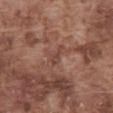biopsy_status: not biopsied; imaged during a skin examination
automated_metrics:
  area_mm2_approx: 3.0
  eccentricity: 0.9
  shape_asymmetry: 0.5
  border_irregularity_0_10: 6.0
  color_variation_0_10: 1.0
  peripheral_color_asymmetry: 0.5
  nevus_likeness_0_100: 0
  lesion_detection_confidence_0_100: 75
patient:
  sex: male
  age_approx: 75
image:
  source: total-body photography crop
  field_of_view_mm: 15
site: abdomen
lesion_size:
  long_diameter_mm_approx: 3.0
lighting: white-light